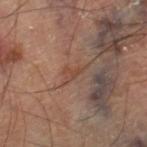| key | value |
|---|---|
| notes | imaged on a skin check; not biopsied |
| size | about 3 mm |
| site | the right thigh |
| imaging modality | ~15 mm crop, total-body skin-cancer survey |